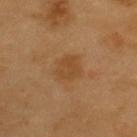biopsy status — catalogued during a skin exam; not biopsied | anatomic site — the upper back | imaging modality — 15 mm crop, total-body photography | patient — male, in their 60s.